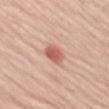workup: imaged on a skin check; not biopsied
automated lesion analysis: an area of roughly 5 mm², an outline eccentricity of about 0.8 (0 = round, 1 = elongated), and a shape-asymmetry score of about 0.15 (0 = symmetric); roughly 12 lightness units darker than nearby skin and a normalized border contrast of about 8
illumination: white-light
subject: male, in their 70s
acquisition: total-body-photography crop, ~15 mm field of view
location: the left thigh